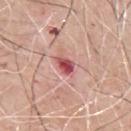Assessment:
No biopsy was performed on this lesion — it was imaged during a full skin examination and was not determined to be concerning.
Background:
On the front of the torso. A male subject aged around 70. An algorithmic analysis of the crop reported an eccentricity of roughly 0.7 and two-axis asymmetry of about 0.3. The analysis additionally found a lesion color around L≈53 a*≈33 b*≈23 in CIELAB, roughly 15 lightness units darker than nearby skin, and a normalized lesion–skin contrast near 10. The software also gave a nevus-likeness score of about 0/100 and a detector confidence of about 100 out of 100 that the crop contains a lesion. A lesion tile, about 15 mm wide, cut from a 3D total-body photograph. About 2.5 mm across.